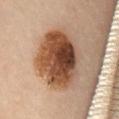Captured during whole-body skin photography for melanoma surveillance; the lesion was not biopsied. Imaged with cross-polarized lighting. An algorithmic analysis of the crop reported a mean CIELAB color near L≈49 a*≈21 b*≈33 and a normalized lesion–skin contrast near 12.5. And it measured a border-irregularity rating of about 1.5/10. It also reported a classifier nevus-likeness of about 60/100. The lesion is on the mid back. A region of skin cropped from a whole-body photographic capture, roughly 15 mm wide. Approximately 7.5 mm at its widest. A female subject, in their mid- to late 50s.